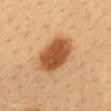workup: total-body-photography surveillance lesion; no biopsy
image source: ~15 mm tile from a whole-body skin photo
automated metrics: an average lesion color of about L≈45 a*≈21 b*≈35 (CIELAB), a lesion–skin lightness drop of about 14, and a lesion-to-skin contrast of about 10.5 (normalized; higher = more distinct); border irregularity of about 1.5 on a 0–10 scale, a color-variation rating of about 3.5/10, and peripheral color asymmetry of about 1
site: the upper back
lighting: cross-polarized illumination
patient: female, aged approximately 40